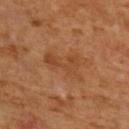<record>
  <biopsy_status>not biopsied; imaged during a skin examination</biopsy_status>
  <lighting>cross-polarized</lighting>
  <patient>
    <sex>male</sex>
    <age_approx>65</age_approx>
  </patient>
  <lesion_size>
    <long_diameter_mm_approx>4.5</long_diameter_mm_approx>
  </lesion_size>
  <image>
    <source>total-body photography crop</source>
    <field_of_view_mm>15</field_of_view_mm>
  </image>
  <site>upper back</site>
</record>